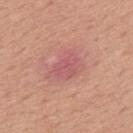Automated tile analysis of the lesion measured a classifier nevus-likeness of about 0/100. The recorded lesion diameter is about 4.5 mm. Imaged with white-light lighting. The lesion is on the upper back. A male subject in their 50s. A close-up tile cropped from a whole-body skin photograph, about 15 mm across.